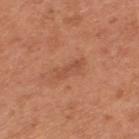Clinical impression:
Imaged during a routine full-body skin examination; the lesion was not biopsied and no histopathology is available.
Acquisition and patient details:
Captured under white-light illumination. A male patient in their mid- to late 60s. The lesion's longest dimension is about 3.5 mm. A 15 mm close-up tile from a total-body photography series done for melanoma screening. Located on the mid back.